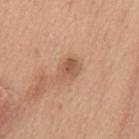Clinical impression: The lesion was tiled from a total-body skin photograph and was not biopsied. Image and clinical context: A female subject, approximately 40 years of age. Captured under white-light illumination. Cropped from a whole-body photographic skin survey; the tile spans about 15 mm. The total-body-photography lesion software estimated a lesion color around L≈56 a*≈21 b*≈32 in CIELAB, a lesion–skin lightness drop of about 10, and a normalized lesion–skin contrast near 6.5. The software also gave border irregularity of about 3.5 on a 0–10 scale, internal color variation of about 3.5 on a 0–10 scale, and a peripheral color-asymmetry measure near 1. The recorded lesion diameter is about 3.5 mm. Located on the mid back.